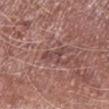{
  "biopsy_status": "not biopsied; imaged during a skin examination",
  "lighting": "white-light",
  "automated_metrics": {
    "area_mm2_approx": 4.0,
    "shape_asymmetry": 0.65,
    "cielab_L": 45,
    "cielab_a": 21,
    "cielab_b": 21,
    "vs_skin_darker_L": 8.0,
    "vs_skin_contrast_norm": 6.0
  },
  "site": "left lower leg",
  "image": {
    "source": "total-body photography crop",
    "field_of_view_mm": 15
  },
  "lesion_size": {
    "long_diameter_mm_approx": 3.5
  },
  "patient": {
    "sex": "male",
    "age_approx": 65
  }
}